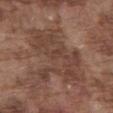workup: no biopsy performed (imaged during a skin exam); acquisition: ~15 mm crop, total-body skin-cancer survey; diameter: ≈7.5 mm; patient: male, roughly 75 years of age; anatomic site: the abdomen.